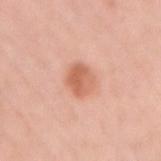No biopsy was performed on this lesion — it was imaged during a full skin examination and was not determined to be concerning.
The lesion is located on the right upper arm.
A female patient roughly 45 years of age.
The lesion-visualizer software estimated a lesion area of about 7.5 mm², an outline eccentricity of about 0.6 (0 = round, 1 = elongated), and a symmetry-axis asymmetry near 0.1. The software also gave a lesion color around L≈63 a*≈26 b*≈34 in CIELAB and a normalized border contrast of about 7.5. The software also gave an automated nevus-likeness rating near 80 out of 100 and a lesion-detection confidence of about 100/100.
A close-up tile cropped from a whole-body skin photograph, about 15 mm across.